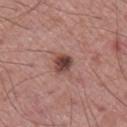<case>
  <biopsy_status>not biopsied; imaged during a skin examination</biopsy_status>
  <image>
    <source>total-body photography crop</source>
    <field_of_view_mm>15</field_of_view_mm>
  </image>
  <automated_metrics>
    <cielab_L>44</cielab_L>
    <cielab_a>22</cielab_a>
    <cielab_b>22</cielab_b>
    <vs_skin_contrast_norm>10.5</vs_skin_contrast_norm>
    <nevus_likeness_0_100>70</nevus_likeness_0_100>
    <lesion_detection_confidence_0_100>100</lesion_detection_confidence_0_100>
  </automated_metrics>
  <patient>
    <sex>male</sex>
    <age_approx>70</age_approx>
  </patient>
  <site>right lower leg</site>
  <lighting>white-light</lighting>
</case>